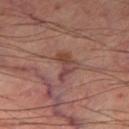Notes:
• workup: no biopsy performed (imaged during a skin exam)
• body site: the leg
• tile lighting: cross-polarized illumination
• diameter: ≈3 mm
• TBP lesion metrics: a nevus-likeness score of about 0/100 and a lesion-detection confidence of about 100/100
• subject: male, roughly 65 years of age
• acquisition: ~15 mm tile from a whole-body skin photo